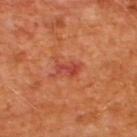| field | value |
|---|---|
| biopsy status | no biopsy performed (imaged during a skin exam) |
| lesion diameter | ≈3 mm |
| tile lighting | cross-polarized illumination |
| location | the upper back |
| image source | total-body-photography crop, ~15 mm field of view |
| subject | male, aged 63 to 67 |